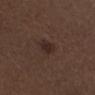tile lighting: white-light illumination
lesion size: about 2.5 mm
imaging modality: ~15 mm crop, total-body skin-cancer survey
location: the right lower leg
patient: male, approximately 50 years of age
TBP lesion metrics: about 6 CIELAB-L* units darker than the surrounding skin and a normalized border contrast of about 7.5; border irregularity of about 2 on a 0–10 scale, a within-lesion color-variation index near 2.5/10, and a peripheral color-asymmetry measure near 1; lesion-presence confidence of about 100/100